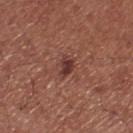Q: Was this lesion biopsied?
A: total-body-photography surveillance lesion; no biopsy
Q: What kind of image is this?
A: ~15 mm crop, total-body skin-cancer survey
Q: How was the tile lit?
A: white-light
Q: Automated lesion metrics?
A: a mean CIELAB color near L≈37 a*≈24 b*≈24, a lesion–skin lightness drop of about 10, and a normalized border contrast of about 9; border irregularity of about 3.5 on a 0–10 scale, a color-variation rating of about 2.5/10, and radial color variation of about 1; a detector confidence of about 100 out of 100 that the crop contains a lesion
Q: Where on the body is the lesion?
A: the right lower leg
Q: Who is the patient?
A: male, aged 68–72
Q: What is the lesion's diameter?
A: about 2.5 mm